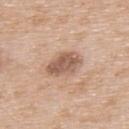No biopsy was performed on this lesion — it was imaged during a full skin examination and was not determined to be concerning.
The lesion is located on the upper back.
A male subject, aged 63 to 67.
A close-up tile cropped from a whole-body skin photograph, about 15 mm across.